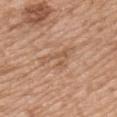No biopsy was performed on this lesion — it was imaged during a full skin examination and was not determined to be concerning. The recorded lesion diameter is about 4.5 mm. The tile uses white-light illumination. A close-up tile cropped from a whole-body skin photograph, about 15 mm across. The patient is a male aged approximately 50. Located on the upper back.